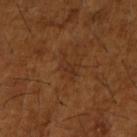The lesion was photographed on a routine skin check and not biopsied; there is no pathology result. A 15 mm close-up extracted from a 3D total-body photography capture. Imaged with cross-polarized lighting. The patient is a male aged 63 to 67. The lesion's longest dimension is about 3 mm. On the right upper arm. The lesion-visualizer software estimated a shape eccentricity near 0.85. The analysis additionally found border irregularity of about 6 on a 0–10 scale and radial color variation of about 0.